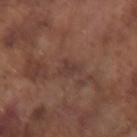| key | value |
|---|---|
| workup | catalogued during a skin exam; not biopsied |
| lighting | white-light illumination |
| lesion diameter | ≈3 mm |
| patient | male, in their mid-70s |
| acquisition | 15 mm crop, total-body photography |
| automated metrics | an area of roughly 3.5 mm², an outline eccentricity of about 0.85 (0 = round, 1 = elongated), and two-axis asymmetry of about 0.35; a lesion–skin lightness drop of about 6 and a lesion-to-skin contrast of about 6 (normalized; higher = more distinct); a border-irregularity index near 3.5/10, internal color variation of about 1 on a 0–10 scale, and peripheral color asymmetry of about 0.5; a classifier nevus-likeness of about 0/100 and a detector confidence of about 85 out of 100 that the crop contains a lesion |
| body site | the right forearm |